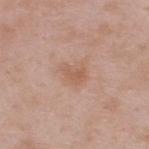<record>
<lighting>white-light</lighting>
<lesion_size>
  <long_diameter_mm_approx>2.5</long_diameter_mm_approx>
</lesion_size>
<image>
  <source>total-body photography crop</source>
  <field_of_view_mm>15</field_of_view_mm>
</image>
<patient>
  <sex>male</sex>
  <age_approx>55</age_approx>
</patient>
<site>upper back</site>
</record>